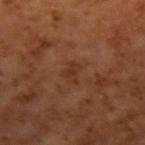{"biopsy_status": "not biopsied; imaged during a skin examination", "lesion_size": {"long_diameter_mm_approx": 2.5}, "patient": {"sex": "male", "age_approx": 60}, "image": {"source": "total-body photography crop", "field_of_view_mm": 15}, "lighting": "cross-polarized", "automated_metrics": {"vs_skin_darker_L": 5.0, "vs_skin_contrast_norm": 5.5, "border_irregularity_0_10": 4.0, "color_variation_0_10": 1.5, "peripheral_color_asymmetry": 0.5}, "site": "arm"}